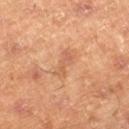The lesion was photographed on a routine skin check and not biopsied; there is no pathology result. A male patient approximately 45 years of age. Automated tile analysis of the lesion measured a border-irregularity index near 4.5/10, a within-lesion color-variation index near 0.5/10, and a peripheral color-asymmetry measure near 0. It also reported a detector confidence of about 100 out of 100 that the crop contains a lesion. The recorded lesion diameter is about 3.5 mm. Imaged with cross-polarized lighting. Located on the right lower leg. Cropped from a whole-body photographic skin survey; the tile spans about 15 mm.